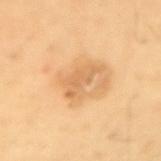Impression: No biopsy was performed on this lesion — it was imaged during a full skin examination and was not determined to be concerning. Acquisition and patient details: Cropped from a whole-body photographic skin survey; the tile spans about 15 mm. The recorded lesion diameter is about 4.5 mm. The patient is a male in their mid- to late 60s. The lesion is located on the right upper arm. Captured under cross-polarized illumination.